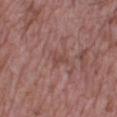Clinical impression: The lesion was photographed on a routine skin check and not biopsied; there is no pathology result. Context: From the right lower leg. A male patient, in their mid- to late 50s. Captured under white-light illumination. The total-body-photography lesion software estimated a mean CIELAB color near L≈46 a*≈22 b*≈23 and a lesion–skin lightness drop of about 6. A 15 mm close-up extracted from a 3D total-body photography capture.